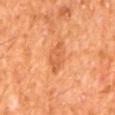Case summary:
• biopsy status — imaged on a skin check; not biopsied
• site — the mid back
• imaging modality — ~15 mm crop, total-body skin-cancer survey
• automated lesion analysis — a lesion area of about 6 mm², a shape eccentricity near 0.9, and a symmetry-axis asymmetry near 0.3; a nevus-likeness score of about 5/100 and a detector confidence of about 100 out of 100 that the crop contains a lesion
• patient — male, aged 63–67
• diameter — ~4.5 mm (longest diameter)
• lighting — cross-polarized illumination